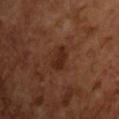biopsy_status: not biopsied; imaged during a skin examination
lighting: cross-polarized
image:
  source: total-body photography crop
  field_of_view_mm: 15
patient:
  sex: male
  age_approx: 65
lesion_size:
  long_diameter_mm_approx: 3.5